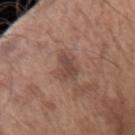• biopsy status — catalogued during a skin exam; not biopsied
• automated metrics — a lesion area of about 6 mm² and a shape eccentricity near 0.85; roughly 9 lightness units darker than nearby skin and a normalized border contrast of about 7.5
• patient — male, in their 70s
• anatomic site — the arm
• diameter — ≈3.5 mm
• tile lighting — white-light illumination
• image — ~15 mm crop, total-body skin-cancer survey